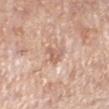The lesion was tiled from a total-body skin photograph and was not biopsied. An algorithmic analysis of the crop reported an eccentricity of roughly 0.8 and a shape-asymmetry score of about 0.5 (0 = symmetric). It also reported a lesion color around L≈62 a*≈21 b*≈29 in CIELAB, a lesion–skin lightness drop of about 9, and a lesion-to-skin contrast of about 6 (normalized; higher = more distinct). The tile uses white-light illumination. This image is a 15 mm lesion crop taken from a total-body photograph. Located on the left lower leg. Longest diameter approximately 3 mm. A female subject about 70 years old.